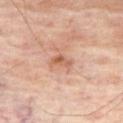Findings:
• notes · imaged on a skin check; not biopsied
• acquisition · ~15 mm tile from a whole-body skin photo
• site · the right thigh
• lesion size · about 2.5 mm
• subject · male, about 70 years old
• tile lighting · cross-polarized illumination
• automated metrics · a lesion area of about 2.5 mm², a shape eccentricity near 0.85, and two-axis asymmetry of about 0.4; an average lesion color of about L≈57 a*≈23 b*≈32 (CIELAB) and a lesion–skin lightness drop of about 10; a border-irregularity index near 3.5/10